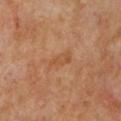Q: Was this lesion biopsied?
A: no biopsy performed (imaged during a skin exam)
Q: What are the patient's age and sex?
A: female, aged around 55
Q: How was the tile lit?
A: cross-polarized illumination
Q: What is the imaging modality?
A: ~15 mm tile from a whole-body skin photo
Q: What did automated image analysis measure?
A: a footprint of about 4 mm², an eccentricity of roughly 0.9, and a shape-asymmetry score of about 0.3 (0 = symmetric); a mean CIELAB color near L≈53 a*≈24 b*≈38, about 6 CIELAB-L* units darker than the surrounding skin, and a normalized lesion–skin contrast near 5.5; a border-irregularity rating of about 3.5/10, a within-lesion color-variation index near 1/10, and radial color variation of about 0
Q: Lesion location?
A: the front of the torso